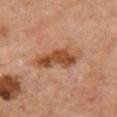The lesion was tiled from a total-body skin photograph and was not biopsied.